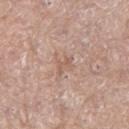biopsy status: total-body-photography surveillance lesion; no biopsy | TBP lesion metrics: an area of roughly 2.5 mm², an eccentricity of roughly 0.9, and a symmetry-axis asymmetry near 0.45; border irregularity of about 5 on a 0–10 scale, a color-variation rating of about 0/10, and a peripheral color-asymmetry measure near 0 | lighting: white-light illumination | acquisition: total-body-photography crop, ~15 mm field of view | body site: the left thigh | subject: female, in their mid-60s | lesion diameter: ~2.5 mm (longest diameter).